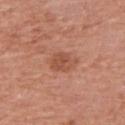Impression: Imaged during a routine full-body skin examination; the lesion was not biopsied and no histopathology is available. Clinical summary: A female subject, aged around 60. A region of skin cropped from a whole-body photographic capture, roughly 15 mm wide. The total-body-photography lesion software estimated a nevus-likeness score of about 50/100 and a detector confidence of about 100 out of 100 that the crop contains a lesion. The recorded lesion diameter is about 3 mm. On the right upper arm.